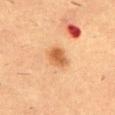Clinical impression: Imaged during a routine full-body skin examination; the lesion was not biopsied and no histopathology is available. Background: This is a cross-polarized tile. A male patient, aged 53–57. On the back. This image is a 15 mm lesion crop taken from a total-body photograph.